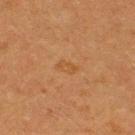Assessment: Part of a total-body skin-imaging series; this lesion was reviewed on a skin check and was not flagged for biopsy. Clinical summary: Measured at roughly 2.5 mm in maximum diameter. Captured under cross-polarized illumination. A 15 mm close-up tile from a total-body photography series done for melanoma screening. A male patient roughly 40 years of age. On the upper back. An algorithmic analysis of the crop reported a footprint of about 3 mm² and a symmetry-axis asymmetry near 0.35. The analysis additionally found a mean CIELAB color near L≈42 a*≈19 b*≈34, roughly 4 lightness units darker than nearby skin, and a normalized lesion–skin contrast near 5. And it measured a border-irregularity rating of about 3/10 and internal color variation of about 0.5 on a 0–10 scale. The analysis additionally found a nevus-likeness score of about 5/100 and lesion-presence confidence of about 100/100.